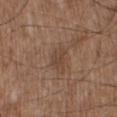notes — total-body-photography surveillance lesion; no biopsy | location — the right lower leg | subject — male, aged 53 to 57 | illumination — white-light | lesion diameter — ~2.5 mm (longest diameter) | image — total-body-photography crop, ~15 mm field of view.